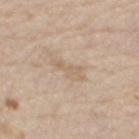biopsy status: total-body-photography surveillance lesion; no biopsy | lighting: white-light illumination | body site: the leg | acquisition: 15 mm crop, total-body photography | TBP lesion metrics: a footprint of about 4 mm², an outline eccentricity of about 0.95 (0 = round, 1 = elongated), and two-axis asymmetry of about 0.45; a lesion color around L≈63 a*≈14 b*≈30 in CIELAB and a normalized lesion–skin contrast near 4.5; a nevus-likeness score of about 0/100 and a lesion-detection confidence of about 90/100 | subject: male, aged 68 to 72.